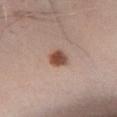{
  "biopsy_status": "not biopsied; imaged during a skin examination",
  "site": "right lower leg",
  "automated_metrics": {
    "area_mm2_approx": 5.0,
    "eccentricity": 0.6,
    "shape_asymmetry": 0.2
  },
  "patient": {
    "sex": "male",
    "age_approx": 25
  },
  "lesion_size": {
    "long_diameter_mm_approx": 3.0
  },
  "image": {
    "source": "total-body photography crop",
    "field_of_view_mm": 15
  },
  "lighting": "white-light"
}